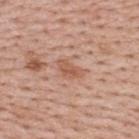Assessment: The lesion was photographed on a routine skin check and not biopsied; there is no pathology result. Image and clinical context: Imaged with white-light lighting. The lesion-visualizer software estimated a footprint of about 4.5 mm², an outline eccentricity of about 0.85 (0 = round, 1 = elongated), and a shape-asymmetry score of about 0.4 (0 = symmetric). The recorded lesion diameter is about 3.5 mm. A male subject approximately 40 years of age. A 15 mm close-up tile from a total-body photography series done for melanoma screening. From the upper back.